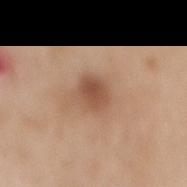* notes — no biopsy performed (imaged during a skin exam)
* site — the mid back
* image — total-body-photography crop, ~15 mm field of view
* subject — female, aged 68–72